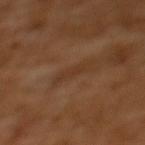Assessment:
The lesion was photographed on a routine skin check and not biopsied; there is no pathology result.
Clinical summary:
A region of skin cropped from a whole-body photographic capture, roughly 15 mm wide. On the back. Imaged with cross-polarized lighting. The lesion's longest dimension is about 3.5 mm. A female subject, in their mid-50s.